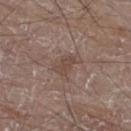biopsy status: no biopsy performed (imaged during a skin exam) | subject: male, approximately 80 years of age | location: the leg | image source: total-body-photography crop, ~15 mm field of view | lesion size: about 3 mm.